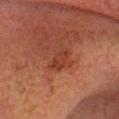Clinical impression:
Imaged during a routine full-body skin examination; the lesion was not biopsied and no histopathology is available.
Acquisition and patient details:
Cropped from a whole-body photographic skin survey; the tile spans about 15 mm. About 3 mm across. Captured under cross-polarized illumination. A male patient in their mid- to late 40s. The lesion is on the head or neck.